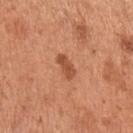| field | value |
|---|---|
| workup | total-body-photography surveillance lesion; no biopsy |
| subject | female, aged approximately 30 |
| image | ~15 mm tile from a whole-body skin photo |
| image-analysis metrics | a mean CIELAB color near L≈52 a*≈27 b*≈36, roughly 11 lightness units darker than nearby skin, and a normalized lesion–skin contrast near 7.5 |
| lesion size | about 3 mm |
| site | the left upper arm |
| lighting | white-light illumination |